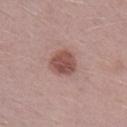The lesion was photographed on a routine skin check and not biopsied; there is no pathology result. The lesion-visualizer software estimated a lesion-to-skin contrast of about 9 (normalized; higher = more distinct). This is a white-light tile. From the right lower leg. Measured at roughly 3.5 mm in maximum diameter. The subject is a male roughly 40 years of age. A 15 mm close-up tile from a total-body photography series done for melanoma screening.